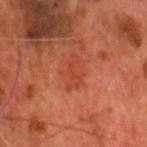The lesion was photographed on a routine skin check and not biopsied; there is no pathology result.
Located on the head or neck.
Imaged with cross-polarized lighting.
Cropped from a whole-body photographic skin survey; the tile spans about 15 mm.
A male patient, aged approximately 70.
The total-body-photography lesion software estimated a lesion color around L≈44 a*≈33 b*≈36 in CIELAB, a lesion–skin lightness drop of about 6, and a normalized lesion–skin contrast near 5. And it measured a border-irregularity rating of about 7.5/10. And it measured a classifier nevus-likeness of about 10/100 and a lesion-detection confidence of about 100/100.